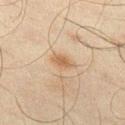Q: Was a biopsy performed?
A: imaged on a skin check; not biopsied
Q: What kind of image is this?
A: total-body-photography crop, ~15 mm field of view
Q: Lesion size?
A: ~3 mm (longest diameter)
Q: What lighting was used for the tile?
A: cross-polarized illumination
Q: What is the anatomic site?
A: the left thigh
Q: Patient demographics?
A: male, aged approximately 45